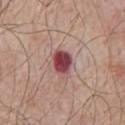No biopsy was performed on this lesion — it was imaged during a full skin examination and was not determined to be concerning.
A male subject, aged 63 to 67.
From the chest.
A roughly 15 mm field-of-view crop from a total-body skin photograph.
This is a white-light tile.
The total-body-photography lesion software estimated a nevus-likeness score of about 0/100 and a detector confidence of about 100 out of 100 that the crop contains a lesion.